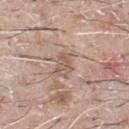Recorded during total-body skin imaging; not selected for excision or biopsy. A male subject, in their mid- to late 60s. From the chest. A roughly 15 mm field-of-view crop from a total-body skin photograph. The lesion's longest dimension is about 3 mm. The tile uses white-light illumination.